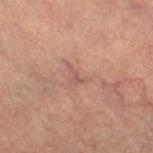This lesion was catalogued during total-body skin photography and was not selected for biopsy. This is a cross-polarized tile. Approximately 3 mm at its widest. A female patient, in their 60s. This image is a 15 mm lesion crop taken from a total-body photograph. On the right thigh.